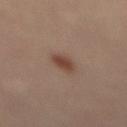Imaged during a routine full-body skin examination; the lesion was not biopsied and no histopathology is available.
The lesion is located on the mid back.
The patient is a male in their mid- to late 60s.
This image is a 15 mm lesion crop taken from a total-body photograph.
About 3 mm across.
The tile uses cross-polarized illumination.